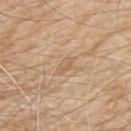The lesion is located on the left upper arm. A 15 mm close-up tile from a total-body photography series done for melanoma screening. The patient is a male aged 78–82.